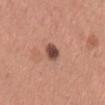{
  "biopsy_status": "not biopsied; imaged during a skin examination",
  "site": "leg",
  "automated_metrics": {
    "area_mm2_approx": 4.0,
    "eccentricity": 0.65,
    "shape_asymmetry": 0.25,
    "cielab_L": 47,
    "cielab_a": 22,
    "cielab_b": 26,
    "vs_skin_darker_L": 16.0,
    "color_variation_0_10": 3.0,
    "peripheral_color_asymmetry": 0.5
  },
  "patient": {
    "sex": "female",
    "age_approx": 30
  },
  "image": {
    "source": "total-body photography crop",
    "field_of_view_mm": 15
  },
  "lesion_size": {
    "long_diameter_mm_approx": 2.5
  },
  "lighting": "white-light"
}